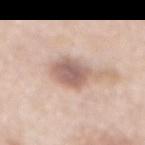Assessment:
The lesion was photographed on a routine skin check and not biopsied; there is no pathology result.
Context:
A roughly 15 mm field-of-view crop from a total-body skin photograph. A female subject approximately 65 years of age. The lesion-visualizer software estimated an eccentricity of roughly 0.5 and a shape-asymmetry score of about 0.15 (0 = symmetric). And it measured a border-irregularity index near 2/10 and a within-lesion color-variation index near 3.5/10. About 4 mm across. From the mid back.